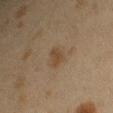The lesion was photographed on a routine skin check and not biopsied; there is no pathology result.
From the right upper arm.
Approximately 3 mm at its widest.
The tile uses cross-polarized illumination.
A female subject, roughly 40 years of age.
A 15 mm crop from a total-body photograph taken for skin-cancer surveillance.